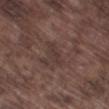Q: Illumination type?
A: white-light illumination
Q: What is the imaging modality?
A: total-body-photography crop, ~15 mm field of view
Q: Patient demographics?
A: male, roughly 75 years of age
Q: Where on the body is the lesion?
A: the left thigh
Q: How large is the lesion?
A: about 3.5 mm